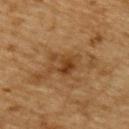Q: Was this lesion biopsied?
A: catalogued during a skin exam; not biopsied
Q: Who is the patient?
A: male, aged around 85 or older
Q: Lesion size?
A: about 3.5 mm
Q: What is the imaging modality?
A: ~15 mm tile from a whole-body skin photo
Q: Where on the body is the lesion?
A: the upper back